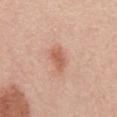Assessment:
Captured during whole-body skin photography for melanoma surveillance; the lesion was not biopsied.
Background:
Cropped from a whole-body photographic skin survey; the tile spans about 15 mm. The patient is a male roughly 65 years of age. Measured at roughly 3 mm in maximum diameter. The lesion is on the mid back. Captured under white-light illumination.